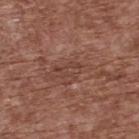| key | value |
|---|---|
| diameter | ≈3 mm |
| site | the upper back |
| image source | ~15 mm crop, total-body skin-cancer survey |
| illumination | white-light |
| patient | male, aged around 75 |